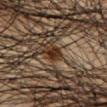Q: Is there a histopathology result?
A: total-body-photography surveillance lesion; no biopsy
Q: What kind of image is this?
A: ~15 mm tile from a whole-body skin photo
Q: What are the patient's age and sex?
A: male, in their 50s
Q: Where on the body is the lesion?
A: the abdomen